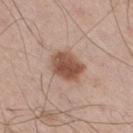Impression: Part of a total-body skin-imaging series; this lesion was reviewed on a skin check and was not flagged for biopsy. Background: The subject is a male roughly 60 years of age. A close-up tile cropped from a whole-body skin photograph, about 15 mm across. On the right thigh.